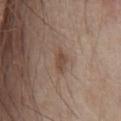Part of a total-body skin-imaging series; this lesion was reviewed on a skin check and was not flagged for biopsy.